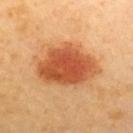Assessment:
Captured during whole-body skin photography for melanoma surveillance; the lesion was not biopsied.
Image and clinical context:
The lesion is on the upper back. The subject is a female about 60 years old. The tile uses cross-polarized illumination. A 15 mm crop from a total-body photograph taken for skin-cancer surveillance. About 7.5 mm across.